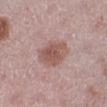Findings:
* notes: imaged on a skin check; not biopsied
* location: the left lower leg
* patient: female, aged approximately 45
* acquisition: total-body-photography crop, ~15 mm field of view
* tile lighting: white-light illumination